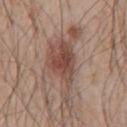Q: Was a biopsy performed?
A: no biopsy performed (imaged during a skin exam)
Q: What lighting was used for the tile?
A: white-light illumination
Q: What are the patient's age and sex?
A: male, aged 53–57
Q: Where on the body is the lesion?
A: the chest
Q: How was this image acquired?
A: 15 mm crop, total-body photography